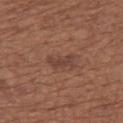Part of a total-body skin-imaging series; this lesion was reviewed on a skin check and was not flagged for biopsy. Imaged with white-light lighting. Longest diameter approximately 3 mm. The lesion is on the left thigh. Cropped from a total-body skin-imaging series; the visible field is about 15 mm. The subject is a female about 55 years old. Automated image analysis of the tile measured a color-variation rating of about 1/10 and radial color variation of about 0.